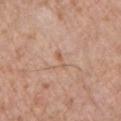Q: Was this lesion biopsied?
A: no biopsy performed (imaged during a skin exam)
Q: How was the tile lit?
A: white-light illumination
Q: How large is the lesion?
A: about 3 mm
Q: Where on the body is the lesion?
A: the left upper arm
Q: What is the imaging modality?
A: total-body-photography crop, ~15 mm field of view
Q: What are the patient's age and sex?
A: male, aged around 55